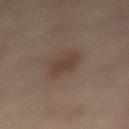<lesion>
  <biopsy_status>not biopsied; imaged during a skin examination</biopsy_status>
  <image>
    <source>total-body photography crop</source>
    <field_of_view_mm>15</field_of_view_mm>
  </image>
  <site>mid back</site>
  <patient>
    <sex>female</sex>
    <age_approx>60</age_approx>
  </patient>
</lesion>